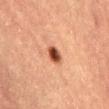Clinical impression:
No biopsy was performed on this lesion — it was imaged during a full skin examination and was not determined to be concerning.
Image and clinical context:
The patient is a female roughly 70 years of age. From the abdomen. A region of skin cropped from a whole-body photographic capture, roughly 15 mm wide. The recorded lesion diameter is about 2.5 mm. Automated image analysis of the tile measured an average lesion color of about L≈42 a*≈24 b*≈30 (CIELAB), a lesion–skin lightness drop of about 17, and a normalized border contrast of about 12.5. It also reported a classifier nevus-likeness of about 100/100 and a lesion-detection confidence of about 100/100.